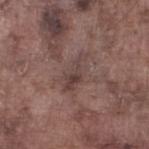Captured during whole-body skin photography for melanoma surveillance; the lesion was not biopsied.